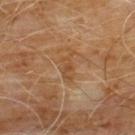Captured during whole-body skin photography for melanoma surveillance; the lesion was not biopsied. Measured at roughly 3.5 mm in maximum diameter. An algorithmic analysis of the crop reported a lesion area of about 4.5 mm², an eccentricity of roughly 0.9, and a shape-asymmetry score of about 0.4 (0 = symmetric). The analysis additionally found a border-irregularity rating of about 5/10, a within-lesion color-variation index near 1.5/10, and peripheral color asymmetry of about 0.5. A male subject, approximately 60 years of age. Cropped from a whole-body photographic skin survey; the tile spans about 15 mm. Located on the front of the torso.